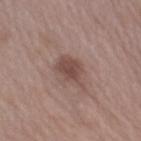The lesion was tiled from a total-body skin photograph and was not biopsied.
Automated image analysis of the tile measured a border-irregularity rating of about 3.5/10, internal color variation of about 1.5 on a 0–10 scale, and a peripheral color-asymmetry measure near 0.5.
The lesion is on the right upper arm.
A 15 mm crop from a total-body photograph taken for skin-cancer surveillance.
A female patient roughly 70 years of age.
Approximately 4.5 mm at its widest.
This is a white-light tile.